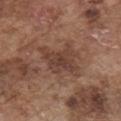Assessment:
Part of a total-body skin-imaging series; this lesion was reviewed on a skin check and was not flagged for biopsy.
Acquisition and patient details:
Cropped from a whole-body photographic skin survey; the tile spans about 15 mm. A male subject in their mid- to late 70s. The lesion is on the chest.